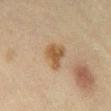<lesion>
<biopsy_status>not biopsied; imaged during a skin examination</biopsy_status>
<image>
  <source>total-body photography crop</source>
  <field_of_view_mm>15</field_of_view_mm>
</image>
<patient>
  <sex>female</sex>
  <age_approx>65</age_approx>
</patient>
<lighting>cross-polarized</lighting>
<site>right lower leg</site>
<lesion_size>
  <long_diameter_mm_approx>3.5</long_diameter_mm_approx>
</lesion_size>
</lesion>